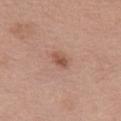The lesion was tiled from a total-body skin photograph and was not biopsied. A female subject about 65 years old. Measured at roughly 2.5 mm in maximum diameter. A roughly 15 mm field-of-view crop from a total-body skin photograph. From the chest.